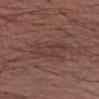This lesion was catalogued during total-body skin photography and was not selected for biopsy. From the left forearm. A male patient, aged around 70. A 15 mm close-up extracted from a 3D total-body photography capture.